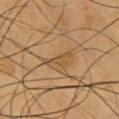Q: Was this lesion biopsied?
A: no biopsy performed (imaged during a skin exam)
Q: Lesion location?
A: the chest
Q: Patient demographics?
A: male, aged 58 to 62
Q: How was this image acquired?
A: ~15 mm crop, total-body skin-cancer survey
Q: Lesion size?
A: about 3.5 mm
Q: How was the tile lit?
A: cross-polarized